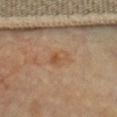workup — no biopsy performed (imaged during a skin exam)
location — the chest
diameter — ~3 mm (longest diameter)
tile lighting — cross-polarized illumination
patient — female, in their 60s
automated lesion analysis — a lesion–skin lightness drop of about 6 and a normalized border contrast of about 6; a classifier nevus-likeness of about 0/100
imaging modality — total-body-photography crop, ~15 mm field of view